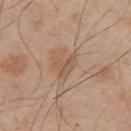notes: catalogued during a skin exam; not biopsied | lesion diameter: about 3 mm | anatomic site: the upper back | subject: male, roughly 55 years of age | illumination: white-light illumination | automated lesion analysis: a shape eccentricity near 0.6 and a shape-asymmetry score of about 0.55 (0 = symmetric); a border-irregularity rating of about 5.5/10 and a within-lesion color-variation index near 1/10 | acquisition: ~15 mm crop, total-body skin-cancer survey.